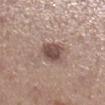{"image": {"source": "total-body photography crop", "field_of_view_mm": 15}, "site": "right lower leg", "automated_metrics": {"area_mm2_approx": 6.5, "shape_asymmetry": 0.2, "border_irregularity_0_10": 2.0, "color_variation_0_10": 3.0, "peripheral_color_asymmetry": 1.0, "nevus_likeness_0_100": 60, "lesion_detection_confidence_0_100": 100}, "lighting": "white-light", "patient": {"sex": "female", "age_approx": 40}, "lesion_size": {"long_diameter_mm_approx": 3.5}}